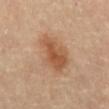The lesion was tiled from a total-body skin photograph and was not biopsied. The subject is a male aged around 85 or older. From the front of the torso. A 15 mm close-up tile from a total-body photography series done for melanoma screening. An algorithmic analysis of the crop reported an eccentricity of roughly 0.85 and a shape-asymmetry score of about 0.25 (0 = symmetric). It also reported an average lesion color of about L≈51 a*≈21 b*≈33 (CIELAB), about 10 CIELAB-L* units darker than the surrounding skin, and a lesion-to-skin contrast of about 7.5 (normalized; higher = more distinct). The software also gave an automated nevus-likeness rating near 90 out of 100 and lesion-presence confidence of about 100/100. Captured under cross-polarized illumination.